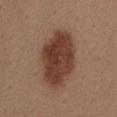Q: Was this lesion biopsied?
A: imaged on a skin check; not biopsied
Q: How was this image acquired?
A: total-body-photography crop, ~15 mm field of view
Q: Where on the body is the lesion?
A: the abdomen
Q: Who is the patient?
A: female, aged around 40
Q: Lesion size?
A: about 7.5 mm
Q: Automated lesion metrics?
A: a border-irregularity rating of about 1.5/10, internal color variation of about 4 on a 0–10 scale, and a peripheral color-asymmetry measure near 1.5; a lesion-detection confidence of about 100/100